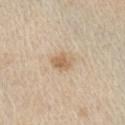Imaged during a routine full-body skin examination; the lesion was not biopsied and no histopathology is available. The subject is a male approximately 60 years of age. On the right upper arm. Automated tile analysis of the lesion measured about 10 CIELAB-L* units darker than the surrounding skin and a normalized lesion–skin contrast near 7. The software also gave lesion-presence confidence of about 100/100. A 15 mm close-up tile from a total-body photography series done for melanoma screening. This is a white-light tile. About 3 mm across.